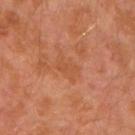lesion size: ~2.5 mm (longest diameter) | location: the left arm | subject: male, aged 28–32 | lighting: cross-polarized | imaging modality: total-body-photography crop, ~15 mm field of view | automated lesion analysis: an area of roughly 4 mm² and a shape eccentricity near 0.7; a mean CIELAB color near L≈50 a*≈26 b*≈37, roughly 5 lightness units darker than nearby skin, and a lesion-to-skin contrast of about 4.5 (normalized; higher = more distinct); a border-irregularity index near 4.5/10, internal color variation of about 1 on a 0–10 scale, and peripheral color asymmetry of about 0.5.